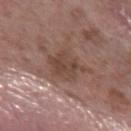| feature | finding |
|---|---|
| workup | catalogued during a skin exam; not biopsied |
| image source | ~15 mm crop, total-body skin-cancer survey |
| subject | male, aged 58–62 |
| anatomic site | the left forearm |
| tile lighting | white-light illumination |